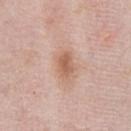Clinical impression:
Imaged during a routine full-body skin examination; the lesion was not biopsied and no histopathology is available.
Image and clinical context:
A female patient in their 60s. A lesion tile, about 15 mm wide, cut from a 3D total-body photograph. From the chest. The lesion-visualizer software estimated a lesion color around L≈60 a*≈21 b*≈30 in CIELAB, roughly 10 lightness units darker than nearby skin, and a lesion-to-skin contrast of about 7.5 (normalized; higher = more distinct). The recorded lesion diameter is about 3.5 mm.